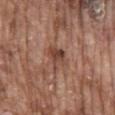biopsy_status: not biopsied; imaged during a skin examination
site: mid back
automated_metrics:
  area_mm2_approx: 6.0
  shape_asymmetry: 0.35
  cielab_L: 44
  cielab_a: 21
  cielab_b: 27
  vs_skin_darker_L: 10.0
  vs_skin_contrast_norm: 7.5
image:
  source: total-body photography crop
  field_of_view_mm: 15
patient:
  sex: male
  age_approx: 75
lighting: white-light
lesion_size:
  long_diameter_mm_approx: 3.0